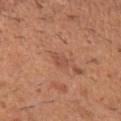- notes — catalogued during a skin exam; not biopsied
- image — 15 mm crop, total-body photography
- location — the left upper arm
- subject — male, aged 38 to 42